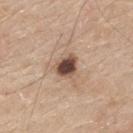The lesion was tiled from a total-body skin photograph and was not biopsied. An algorithmic analysis of the crop reported an average lesion color of about L≈47 a*≈18 b*≈25 (CIELAB), about 18 CIELAB-L* units darker than the surrounding skin, and a normalized lesion–skin contrast near 12.5. A male patient, aged 78–82. The recorded lesion diameter is about 3 mm. Located on the mid back. A 15 mm close-up extracted from a 3D total-body photography capture.